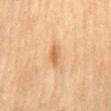Q: Was a biopsy performed?
A: catalogued during a skin exam; not biopsied
Q: How was this image acquired?
A: ~15 mm tile from a whole-body skin photo
Q: Where on the body is the lesion?
A: the mid back
Q: Patient demographics?
A: female, aged around 55